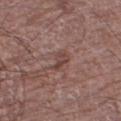No biopsy was performed on this lesion — it was imaged during a full skin examination and was not determined to be concerning. A male subject roughly 75 years of age. Automated tile analysis of the lesion measured border irregularity of about 4 on a 0–10 scale and peripheral color asymmetry of about 0.5. The lesion's longest dimension is about 3 mm. The lesion is located on the right thigh. A 15 mm close-up tile from a total-body photography series done for melanoma screening. The tile uses white-light illumination.